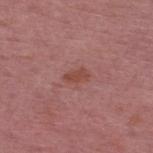biopsy status — imaged on a skin check; not biopsied
image — ~15 mm crop, total-body skin-cancer survey
lesion size — ~2.5 mm (longest diameter)
lighting — white-light illumination
location — the upper back
automated lesion analysis — a lesion area of about 3.5 mm² and a symmetry-axis asymmetry near 0.25; an automated nevus-likeness rating near 0 out of 100 and lesion-presence confidence of about 100/100
patient — male, in their mid-50s